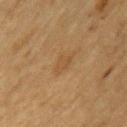{"biopsy_status": "not biopsied; imaged during a skin examination", "site": "arm", "image": {"source": "total-body photography crop", "field_of_view_mm": 15}, "automated_metrics": {"eccentricity": 0.75, "shape_asymmetry": 0.25, "cielab_L": 43, "cielab_a": 16, "cielab_b": 33, "vs_skin_darker_L": 5.0, "vs_skin_contrast_norm": 4.5, "border_irregularity_0_10": 2.5, "color_variation_0_10": 1.0, "nevus_likeness_0_100": 5}, "lighting": "cross-polarized", "patient": {"sex": "male", "age_approx": "85+"}}